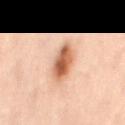workup = no biopsy performed (imaged during a skin exam); subject = female, roughly 55 years of age; imaging modality = ~15 mm tile from a whole-body skin photo; size = about 5 mm; tile lighting = cross-polarized; site = the mid back; automated lesion analysis = a lesion area of about 9 mm², a shape eccentricity near 0.85, and a symmetry-axis asymmetry near 0.25.